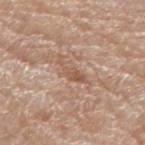notes = catalogued during a skin exam; not biopsied
anatomic site = the left forearm
subject = female, in their mid- to late 70s
TBP lesion metrics = about 9 CIELAB-L* units darker than the surrounding skin and a normalized border contrast of about 6.5; a border-irregularity rating of about 6.5/10 and radial color variation of about 0.5; a nevus-likeness score of about 0/100 and lesion-presence confidence of about 90/100
image source = ~15 mm tile from a whole-body skin photo
illumination = white-light